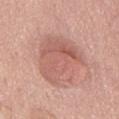follow-up: catalogued during a skin exam; not biopsied | location: the chest | subject: male, aged approximately 75 | automated lesion analysis: a footprint of about 27 mm², an outline eccentricity of about 0.55 (0 = round, 1 = elongated), and a shape-asymmetry score of about 0.25 (0 = symmetric); a border-irregularity index near 3/10, internal color variation of about 5 on a 0–10 scale, and a peripheral color-asymmetry measure near 1.5; a nevus-likeness score of about 30/100 and a detector confidence of about 100 out of 100 that the crop contains a lesion | lesion diameter: ≈7 mm | imaging modality: 15 mm crop, total-body photography.